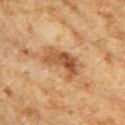notes = catalogued during a skin exam; not biopsied.